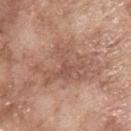Assessment:
Recorded during total-body skin imaging; not selected for excision or biopsy.
Acquisition and patient details:
The recorded lesion diameter is about 7.5 mm. Cropped from a whole-body photographic skin survey; the tile spans about 15 mm. A male subject, in their mid-60s. Imaged with white-light lighting. The lesion is on the upper back. An algorithmic analysis of the crop reported an area of roughly 20 mm². It also reported an average lesion color of about L≈54 a*≈21 b*≈27 (CIELAB), about 9 CIELAB-L* units darker than the surrounding skin, and a normalized border contrast of about 6. The analysis additionally found border irregularity of about 8 on a 0–10 scale, a color-variation rating of about 3.5/10, and peripheral color asymmetry of about 1.5.